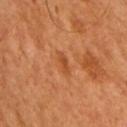Assessment:
This lesion was catalogued during total-body skin photography and was not selected for biopsy.
Image and clinical context:
Cropped from a whole-body photographic skin survey; the tile spans about 15 mm. The patient is a male aged around 50. About 2.5 mm across. The lesion is located on the right upper arm. Captured under cross-polarized illumination.